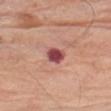workup: catalogued during a skin exam; not biopsied
body site: the arm
subject: male, roughly 80 years of age
diameter: about 2.5 mm
tile lighting: white-light illumination
acquisition: ~15 mm crop, total-body skin-cancer survey